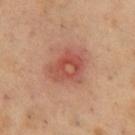Impression: This lesion was catalogued during total-body skin photography and was not selected for biopsy. Context: A 15 mm close-up tile from a total-body photography series done for melanoma screening. A male subject, aged 48–52. The total-body-photography lesion software estimated a lesion color around L≈51 a*≈28 b*≈30 in CIELAB and roughly 9 lightness units darker than nearby skin. It also reported a border-irregularity rating of about 3/10 and peripheral color asymmetry of about 2. And it measured a nevus-likeness score of about 0/100 and a lesion-detection confidence of about 100/100. From the chest. The tile uses cross-polarized illumination.